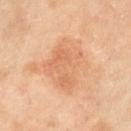Case summary:
– workup: imaged on a skin check; not biopsied
– patient: female, aged approximately 70
– acquisition: ~15 mm tile from a whole-body skin photo
– diameter: ~5.5 mm (longest diameter)
– location: the right lower leg